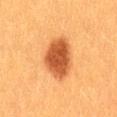Recorded during total-body skin imaging; not selected for excision or biopsy. Measured at roughly 5 mm in maximum diameter. Cropped from a whole-body photographic skin survey; the tile spans about 15 mm. Located on the lower back. The subject is a female aged 38 to 42. An algorithmic analysis of the crop reported an outline eccentricity of about 0.65 (0 = round, 1 = elongated) and a symmetry-axis asymmetry near 0.15. And it measured a lesion color around L≈46 a*≈26 b*≈38 in CIELAB, a lesion–skin lightness drop of about 14, and a normalized lesion–skin contrast near 10. The software also gave a border-irregularity rating of about 1.5/10, a within-lesion color-variation index near 3.5/10, and radial color variation of about 1. And it measured a lesion-detection confidence of about 100/100. The tile uses cross-polarized illumination.